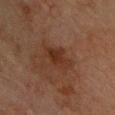Case summary:
• workup · catalogued during a skin exam; not biopsied
• image · ~15 mm crop, total-body skin-cancer survey
• lesion diameter · ≈4 mm
• illumination · cross-polarized
• body site · the chest
• subject · male, roughly 75 years of age